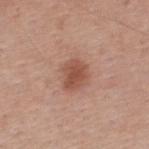A male subject, aged 38–42.
This is a white-light tile.
Automated tile analysis of the lesion measured an outline eccentricity of about 0.6 (0 = round, 1 = elongated) and two-axis asymmetry of about 0.25. It also reported a lesion color around L≈51 a*≈24 b*≈29 in CIELAB, a lesion–skin lightness drop of about 11, and a normalized lesion–skin contrast near 8. And it measured border irregularity of about 2 on a 0–10 scale, internal color variation of about 2.5 on a 0–10 scale, and a peripheral color-asymmetry measure near 0.5. And it measured a nevus-likeness score of about 90/100 and a detector confidence of about 100 out of 100 that the crop contains a lesion.
Cropped from a whole-body photographic skin survey; the tile spans about 15 mm.
The lesion is located on the back.